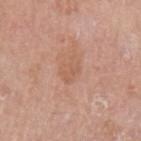Imaged during a routine full-body skin examination; the lesion was not biopsied and no histopathology is available.
The total-body-photography lesion software estimated a mean CIELAB color near L≈58 a*≈21 b*≈31 and a normalized border contrast of about 4.5. It also reported internal color variation of about 2 on a 0–10 scale and peripheral color asymmetry of about 0.5.
A lesion tile, about 15 mm wide, cut from a 3D total-body photograph.
The recorded lesion diameter is about 3 mm.
The lesion is on the left upper arm.
The tile uses white-light illumination.
A male patient, aged 63 to 67.